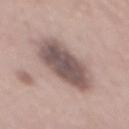Q: Was this lesion biopsied?
A: no biopsy performed (imaged during a skin exam)
Q: Illumination type?
A: white-light illumination
Q: What kind of image is this?
A: ~15 mm crop, total-body skin-cancer survey
Q: Patient demographics?
A: male, roughly 55 years of age
Q: Where on the body is the lesion?
A: the mid back
Q: Lesion size?
A: about 7.5 mm